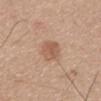This lesion was catalogued during total-body skin photography and was not selected for biopsy.
Longest diameter approximately 4 mm.
Cropped from a total-body skin-imaging series; the visible field is about 15 mm.
The subject is a male aged around 65.
This is a white-light tile.
The lesion is located on the mid back.